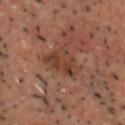The lesion was photographed on a routine skin check and not biopsied; there is no pathology result.
From the head or neck.
A 15 mm close-up extracted from a 3D total-body photography capture.
A male patient, in their 50s.
Approximately 4.5 mm at its widest.
This is a cross-polarized tile.